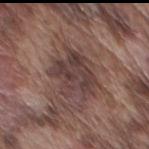Assessment: No biopsy was performed on this lesion — it was imaged during a full skin examination and was not determined to be concerning. Acquisition and patient details: A male subject aged around 75. Located on the front of the torso. A 15 mm crop from a total-body photograph taken for skin-cancer surveillance.